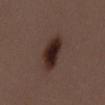| field | value |
|---|---|
| biopsy status | no biopsy performed (imaged during a skin exam) |
| site | the mid back |
| patient | female, approximately 50 years of age |
| imaging modality | 15 mm crop, total-body photography |
| tile lighting | white-light |
| automated lesion analysis | border irregularity of about 2.5 on a 0–10 scale, a color-variation rating of about 5/10, and radial color variation of about 1; a classifier nevus-likeness of about 100/100 and a lesion-detection confidence of about 100/100 |
| size | about 5 mm |